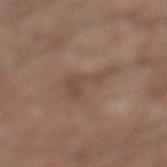Case summary:
- follow-up: no biopsy performed (imaged during a skin exam)
- image source: total-body-photography crop, ~15 mm field of view
- body site: the left lower leg
- image-analysis metrics: roughly 7 lightness units darker than nearby skin and a normalized border contrast of about 5; border irregularity of about 8.5 on a 0–10 scale, a color-variation rating of about 1.5/10, and a peripheral color-asymmetry measure near 0.5
- subject: male, approximately 55 years of age
- tile lighting: white-light
- diameter: about 4.5 mm